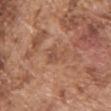| field | value |
|---|---|
| notes | imaged on a skin check; not biopsied |